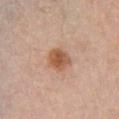notes — no biopsy performed (imaged during a skin exam)
imaging modality — ~15 mm tile from a whole-body skin photo
patient — female, aged 63 to 67
lighting — white-light illumination
diameter — ≈3 mm
anatomic site — the chest
automated metrics — a border-irregularity rating of about 2/10, a color-variation rating of about 4/10, and radial color variation of about 1; an automated nevus-likeness rating near 95 out of 100 and a detector confidence of about 100 out of 100 that the crop contains a lesion